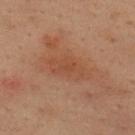Q: Was this lesion biopsied?
A: total-body-photography surveillance lesion; no biopsy
Q: Patient demographics?
A: male, in their mid-30s
Q: What is the anatomic site?
A: the upper back
Q: How was the tile lit?
A: cross-polarized illumination
Q: How was this image acquired?
A: total-body-photography crop, ~15 mm field of view
Q: What is the lesion's diameter?
A: ~5 mm (longest diameter)